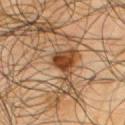Q: How was the tile lit?
A: cross-polarized
Q: What is the lesion's diameter?
A: about 2.5 mm
Q: Lesion location?
A: the back
Q: What is the imaging modality?
A: ~15 mm crop, total-body skin-cancer survey
Q: Automated lesion metrics?
A: border irregularity of about 3 on a 0–10 scale, internal color variation of about 1 on a 0–10 scale, and peripheral color asymmetry of about 0.5
Q: Patient demographics?
A: male, aged 63 to 67